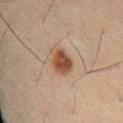Impression:
Imaged during a routine full-body skin examination; the lesion was not biopsied and no histopathology is available.
Background:
Cropped from a whole-body photographic skin survey; the tile spans about 15 mm. An algorithmic analysis of the crop reported roughly 12 lightness units darker than nearby skin and a lesion-to-skin contrast of about 10 (normalized; higher = more distinct). The software also gave a border-irregularity rating of about 1.5/10, internal color variation of about 3.5 on a 0–10 scale, and a peripheral color-asymmetry measure near 1. The analysis additionally found a classifier nevus-likeness of about 100/100 and a detector confidence of about 100 out of 100 that the crop contains a lesion. Imaged with cross-polarized lighting. The subject is a male aged 33 to 37. On the lower back. About 3.5 mm across.